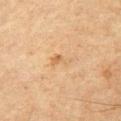notes: total-body-photography surveillance lesion; no biopsy
tile lighting: cross-polarized
subject: male, aged 63 to 67
lesion size: ≈2.5 mm
image-analysis metrics: an area of roughly 3.5 mm², an outline eccentricity of about 0.85 (0 = round, 1 = elongated), and a shape-asymmetry score of about 0.4 (0 = symmetric); a border-irregularity index near 4/10; a nevus-likeness score of about 5/100 and a lesion-detection confidence of about 100/100
body site: the left upper arm
image source: total-body-photography crop, ~15 mm field of view